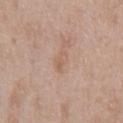biopsy status=no biopsy performed (imaged during a skin exam) | anatomic site=the chest | patient=male, about 50 years old | acquisition=15 mm crop, total-body photography.